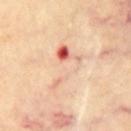Recorded during total-body skin imaging; not selected for excision or biopsy.
On the front of the torso.
A 15 mm crop from a total-body photograph taken for skin-cancer surveillance.
Approximately 6.5 mm at its widest.